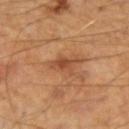workup — imaged on a skin check; not biopsied.